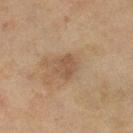No biopsy was performed on this lesion — it was imaged during a full skin examination and was not determined to be concerning.
From the leg.
A female subject aged around 60.
A lesion tile, about 15 mm wide, cut from a 3D total-body photograph.
The lesion's longest dimension is about 2.5 mm.